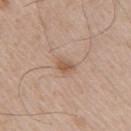A lesion tile, about 15 mm wide, cut from a 3D total-body photograph.
A male subject, aged 63–67.
Imaged with white-light lighting.
The lesion's longest dimension is about 2.5 mm.
The lesion is on the right upper arm.